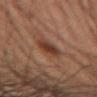Recorded during total-body skin imaging; not selected for excision or biopsy.